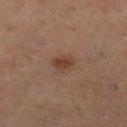workup = no biopsy performed (imaged during a skin exam); imaging modality = ~15 mm crop, total-body skin-cancer survey; patient = female, aged around 55; body site = the leg.